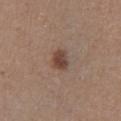This lesion was catalogued during total-body skin photography and was not selected for biopsy. Imaged with white-light lighting. The subject is a male aged approximately 50. The total-body-photography lesion software estimated an average lesion color of about L≈43 a*≈18 b*≈25 (CIELAB), a lesion–skin lightness drop of about 11, and a normalized border contrast of about 9. The analysis additionally found a color-variation rating of about 3/10 and a peripheral color-asymmetry measure near 1. Measured at roughly 2.5 mm in maximum diameter. On the left lower leg. A region of skin cropped from a whole-body photographic capture, roughly 15 mm wide.